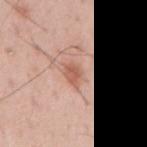{"biopsy_status": "not biopsied; imaged during a skin examination", "site": "back", "image": {"source": "total-body photography crop", "field_of_view_mm": 15}, "lesion_size": {"long_diameter_mm_approx": 3.0}, "patient": {"sex": "male", "age_approx": 55}, "lighting": "white-light", "automated_metrics": {"area_mm2_approx": 4.5, "eccentricity": 0.75, "shape_asymmetry": 0.2, "border_irregularity_0_10": 2.0, "peripheral_color_asymmetry": 0.5}}